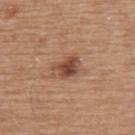Assessment:
This lesion was catalogued during total-body skin photography and was not selected for biopsy.
Image and clinical context:
From the upper back. A male patient, in their mid-60s. A close-up tile cropped from a whole-body skin photograph, about 15 mm across.